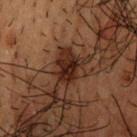Q: Was a biopsy performed?
A: catalogued during a skin exam; not biopsied
Q: Lesion size?
A: ~3.5 mm (longest diameter)
Q: Automated lesion metrics?
A: an area of roughly 5 mm², a shape eccentricity near 0.85, and a symmetry-axis asymmetry near 0.35; border irregularity of about 3.5 on a 0–10 scale, a color-variation rating of about 2.5/10, and a peripheral color-asymmetry measure near 1
Q: Lesion location?
A: the head or neck
Q: What kind of image is this?
A: ~15 mm tile from a whole-body skin photo
Q: Patient demographics?
A: male, aged 48 to 52
Q: Illumination type?
A: cross-polarized